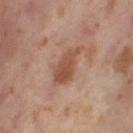Q: Is there a histopathology result?
A: no biopsy performed (imaged during a skin exam)
Q: Lesion location?
A: the left thigh
Q: How was this image acquired?
A: ~15 mm tile from a whole-body skin photo
Q: What are the patient's age and sex?
A: female, about 55 years old